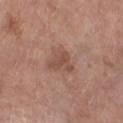Recorded during total-body skin imaging; not selected for excision or biopsy. The lesion is on the left lower leg. The tile uses white-light illumination. A female subject, aged 73 to 77. Automated image analysis of the tile measured a shape eccentricity near 0.75. The software also gave an average lesion color of about L≈49 a*≈20 b*≈26 (CIELAB) and a lesion–skin lightness drop of about 8. A 15 mm close-up tile from a total-body photography series done for melanoma screening. The lesion's longest dimension is about 3.5 mm.